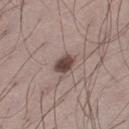{"biopsy_status": "not biopsied; imaged during a skin examination", "lesion_size": {"long_diameter_mm_approx": 2.5}, "automated_metrics": {"area_mm2_approx": 4.5, "eccentricity": 0.65, "shape_asymmetry": 0.15, "cielab_L": 44, "cielab_a": 16, "cielab_b": 20, "vs_skin_darker_L": 15.0, "vs_skin_contrast_norm": 11.0, "color_variation_0_10": 3.5, "peripheral_color_asymmetry": 1.0, "nevus_likeness_0_100": 95, "lesion_detection_confidence_0_100": 100}, "patient": {"sex": "male", "age_approx": 35}, "image": {"source": "total-body photography crop", "field_of_view_mm": 15}, "site": "left thigh"}